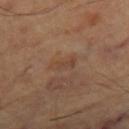Clinical impression: This lesion was catalogued during total-body skin photography and was not selected for biopsy. Acquisition and patient details: Approximately 3 mm at its widest. The lesion is located on the right thigh. This is a cross-polarized tile. The patient is in their mid-60s. Cropped from a total-body skin-imaging series; the visible field is about 15 mm.